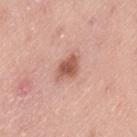This lesion was catalogued during total-body skin photography and was not selected for biopsy.
Longest diameter approximately 3.5 mm.
A lesion tile, about 15 mm wide, cut from a 3D total-body photograph.
On the leg.
A male subject, aged around 50.
Automated image analysis of the tile measured a lesion area of about 6 mm² and a shape-asymmetry score of about 0.25 (0 = symmetric). It also reported a color-variation rating of about 3/10 and peripheral color asymmetry of about 1. The analysis additionally found an automated nevus-likeness rating near 90 out of 100.
This is a white-light tile.